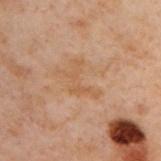Imaged during a routine full-body skin examination; the lesion was not biopsied and no histopathology is available.
This image is a 15 mm lesion crop taken from a total-body photograph.
A male subject, aged 48–52.
Measured at roughly 4.5 mm in maximum diameter.
On the upper back.